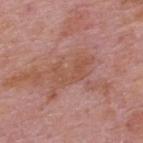Impression:
The lesion was photographed on a routine skin check and not biopsied; there is no pathology result.
Image and clinical context:
A male subject about 75 years old. A region of skin cropped from a whole-body photographic capture, roughly 15 mm wide. On the back.